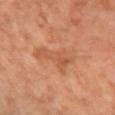Context:
The patient is a female in their mid- to late 60s. From the left forearm. A roughly 15 mm field-of-view crop from a total-body skin photograph. Automated tile analysis of the lesion measured an area of roughly 11 mm², a shape eccentricity near 0.85, and a symmetry-axis asymmetry near 0.5. It also reported an average lesion color of about L≈54 a*≈25 b*≈35 (CIELAB) and roughly 7 lightness units darker than nearby skin. About 6 mm across. The tile uses cross-polarized illumination.